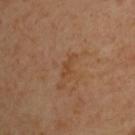Imaged during a routine full-body skin examination; the lesion was not biopsied and no histopathology is available. The subject is a male about 45 years old. A lesion tile, about 15 mm wide, cut from a 3D total-body photograph. The lesion is on the upper back.